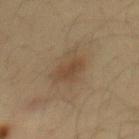The lesion was tiled from a total-body skin photograph and was not biopsied. The subject is a male aged approximately 35. Imaged with cross-polarized lighting. On the mid back. A 15 mm close-up extracted from a 3D total-body photography capture. The recorded lesion diameter is about 5 mm.